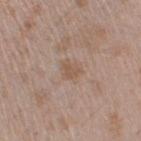Assessment:
Imaged during a routine full-body skin examination; the lesion was not biopsied and no histopathology is available.
Clinical summary:
From the right upper arm. A region of skin cropped from a whole-body photographic capture, roughly 15 mm wide. A male subject about 50 years old.